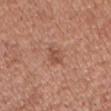| feature | finding |
|---|---|
| workup | total-body-photography surveillance lesion; no biopsy |
| image-analysis metrics | an automated nevus-likeness rating near 0 out of 100 and lesion-presence confidence of about 100/100 |
| anatomic site | the head or neck |
| illumination | white-light |
| lesion size | ≈3 mm |
| image | ~15 mm crop, total-body skin-cancer survey |
| patient | male, about 40 years old |